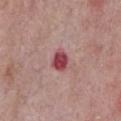Findings:
– workup: imaged on a skin check; not biopsied
– anatomic site: the chest
– TBP lesion metrics: a lesion color around L≈46 a*≈32 b*≈20 in CIELAB, roughly 15 lightness units darker than nearby skin, and a normalized border contrast of about 10.5; a classifier nevus-likeness of about 0/100 and a lesion-detection confidence of about 100/100
– patient: male, aged 63–67
– imaging modality: total-body-photography crop, ~15 mm field of view